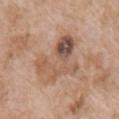Assessment: Part of a total-body skin-imaging series; this lesion was reviewed on a skin check and was not flagged for biopsy. Background: Captured under white-light illumination. A female patient in their mid-70s. Approximately 7 mm at its widest. Automated tile analysis of the lesion measured a lesion area of about 19 mm² and an eccentricity of roughly 0.8. The analysis additionally found internal color variation of about 10 on a 0–10 scale and radial color variation of about 5. The software also gave a classifier nevus-likeness of about 0/100 and a lesion-detection confidence of about 100/100. On the right upper arm. A region of skin cropped from a whole-body photographic capture, roughly 15 mm wide.